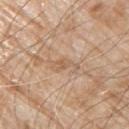Findings:
- follow-up: catalogued during a skin exam; not biopsied
- anatomic site: the right upper arm
- acquisition: 15 mm crop, total-body photography
- tile lighting: white-light
- patient: male, in their 80s
- lesion size: about 3.5 mm